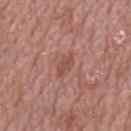Clinical impression:
This lesion was catalogued during total-body skin photography and was not selected for biopsy.
Acquisition and patient details:
This is a white-light tile. Automated tile analysis of the lesion measured a lesion area of about 3.5 mm², a shape eccentricity near 0.85, and a shape-asymmetry score of about 0.3 (0 = symmetric). And it measured an average lesion color of about L≈49 a*≈24 b*≈28 (CIELAB), about 8 CIELAB-L* units darker than the surrounding skin, and a normalized lesion–skin contrast near 6.5. It also reported a border-irregularity index near 3/10 and internal color variation of about 1 on a 0–10 scale. Longest diameter approximately 3 mm. The patient is a male aged around 65. Located on the mid back. Cropped from a whole-body photographic skin survey; the tile spans about 15 mm.